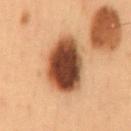Case summary:
• notes: imaged on a skin check; not biopsied
• lighting: cross-polarized
• TBP lesion metrics: a nevus-likeness score of about 90/100 and a lesion-detection confidence of about 100/100
• body site: the mid back
• acquisition: ~15 mm tile from a whole-body skin photo
• patient: male, aged around 55